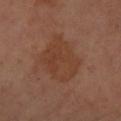  lesion_size:
    long_diameter_mm_approx: 5.5
  site: left forearm
  patient:
    sex: female
    age_approx: 65
  image:
    source: total-body photography crop
    field_of_view_mm: 15
  lighting: cross-polarized
  automated_metrics:
    area_mm2_approx: 20.0
    eccentricity: 0.6
    shape_asymmetry: 0.25
    cielab_L: 39
    cielab_a: 21
    cielab_b: 29
    vs_skin_darker_L: 6.0
    vs_skin_contrast_norm: 6.0